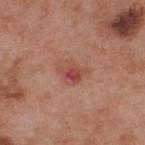Assessment: The lesion was photographed on a routine skin check and not biopsied; there is no pathology result. Background: On the upper back. The total-body-photography lesion software estimated a lesion area of about 5.5 mm² and two-axis asymmetry of about 0.3. And it measured a within-lesion color-variation index near 10/10. Imaged with white-light lighting. A male subject aged 53 to 57. A 15 mm close-up extracted from a 3D total-body photography capture. About 3 mm across.